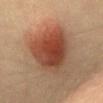workup: no biopsy performed (imaged during a skin exam); diameter: about 7 mm; subject: male, aged around 40; image: ~15 mm tile from a whole-body skin photo; site: the front of the torso; illumination: cross-polarized illumination.